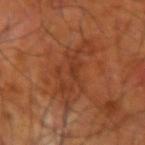  biopsy_status: not biopsied; imaged during a skin examination
  automated_metrics:
    cielab_L: 39
    cielab_a: 26
    cielab_b: 34
    vs_skin_darker_L: 7.0
    vs_skin_contrast_norm: 5.5
    color_variation_0_10: 3.0
    nevus_likeness_0_100: 0
    lesion_detection_confidence_0_100: 100
  lighting: cross-polarized
  image:
    source: total-body photography crop
    field_of_view_mm: 15
  patient:
    sex: male
    age_approx: 65
  site: right upper arm
  lesion_size:
    long_diameter_mm_approx: 7.0